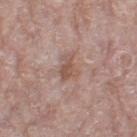patient = female, in their mid-70s | acquisition = ~15 mm crop, total-body skin-cancer survey | lesion diameter = about 3 mm | body site = the left thigh.